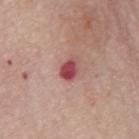Q: Was this lesion biopsied?
A: catalogued during a skin exam; not biopsied
Q: Illumination type?
A: white-light
Q: What is the anatomic site?
A: the mid back
Q: What is the lesion's diameter?
A: about 2.5 mm
Q: What kind of image is this?
A: ~15 mm crop, total-body skin-cancer survey
Q: What are the patient's age and sex?
A: male, aged approximately 75